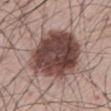Recorded during total-body skin imaging; not selected for excision or biopsy.
A male patient, roughly 65 years of age.
The lesion's longest dimension is about 9.5 mm.
This is a white-light tile.
Located on the mid back.
Automated tile analysis of the lesion measured a shape eccentricity near 0.8. It also reported a normalized border contrast of about 13. And it measured a border-irregularity rating of about 2.5/10, a within-lesion color-variation index near 7/10, and a peripheral color-asymmetry measure near 2.5.
A lesion tile, about 15 mm wide, cut from a 3D total-body photograph.